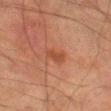Captured during whole-body skin photography for melanoma surveillance; the lesion was not biopsied. The recorded lesion diameter is about 2.5 mm. Cropped from a whole-body photographic skin survey; the tile spans about 15 mm. This is a cross-polarized tile. A male patient aged 78 to 82. The lesion is on the left thigh.